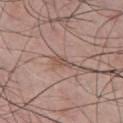Assessment:
The lesion was photographed on a routine skin check and not biopsied; there is no pathology result.
Context:
A male patient, aged around 45. A 15 mm close-up extracted from a 3D total-body photography capture. Automated image analysis of the tile measured an area of roughly 2.5 mm², a shape eccentricity near 0.9, and a shape-asymmetry score of about 0.3 (0 = symmetric). And it measured about 8 CIELAB-L* units darker than the surrounding skin and a normalized lesion–skin contrast near 6. The software also gave a border-irregularity rating of about 3/10. And it measured an automated nevus-likeness rating near 0 out of 100 and a detector confidence of about 95 out of 100 that the crop contains a lesion. Captured under white-light illumination. The lesion is on the chest.